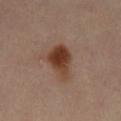Captured during whole-body skin photography for melanoma surveillance; the lesion was not biopsied. The lesion is located on the abdomen. A female subject, roughly 40 years of age. A 15 mm close-up tile from a total-body photography series done for melanoma screening.